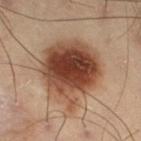notes — no biopsy performed (imaged during a skin exam) | site — the right thigh | automated lesion analysis — an average lesion color of about L≈35 a*≈18 b*≈25 (CIELAB), a lesion–skin lightness drop of about 15, and a lesion-to-skin contrast of about 12.5 (normalized; higher = more distinct); an automated nevus-likeness rating near 100 out of 100 and a lesion-detection confidence of about 100/100 | patient — male, roughly 55 years of age | lesion diameter — ≈7.5 mm | acquisition — ~15 mm tile from a whole-body skin photo.